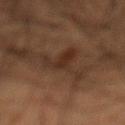– follow-up — no biopsy performed (imaged during a skin exam)
– illumination — cross-polarized
– site — the abdomen
– size — ≈7 mm
– image source — total-body-photography crop, ~15 mm field of view
– patient — male, aged 53–57